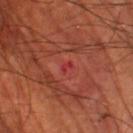Part of a total-body skin-imaging series; this lesion was reviewed on a skin check and was not flagged for biopsy.
The lesion's longest dimension is about 1.5 mm.
A male subject, roughly 70 years of age.
The tile uses cross-polarized illumination.
A 15 mm crop from a total-body photograph taken for skin-cancer surveillance.
On the left lower leg.